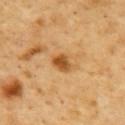The lesion was photographed on a routine skin check and not biopsied; there is no pathology result. Captured under cross-polarized illumination. A 15 mm close-up tile from a total-body photography series done for melanoma screening. On the back. The lesion's longest dimension is about 3 mm. A male patient, aged around 50.